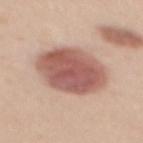Q: Was a biopsy performed?
A: total-body-photography surveillance lesion; no biopsy
Q: Who is the patient?
A: female, aged around 45
Q: Illumination type?
A: white-light
Q: Lesion location?
A: the mid back
Q: What is the imaging modality?
A: ~15 mm crop, total-body skin-cancer survey
Q: What is the lesion's diameter?
A: ~6.5 mm (longest diameter)